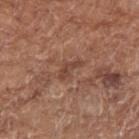The lesion was photographed on a routine skin check and not biopsied; there is no pathology result. A female subject about 75 years old. Imaged with white-light lighting. The total-body-photography lesion software estimated a lesion color around L≈44 a*≈22 b*≈27 in CIELAB. The analysis additionally found a border-irregularity index near 5/10 and peripheral color asymmetry of about 0. And it measured a classifier nevus-likeness of about 0/100 and a lesion-detection confidence of about 90/100. The lesion is on the right forearm. This image is a 15 mm lesion crop taken from a total-body photograph.